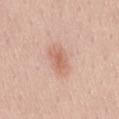No biopsy was performed on this lesion — it was imaged during a full skin examination and was not determined to be concerning. From the lower back. Captured under white-light illumination. A 15 mm close-up extracted from a 3D total-body photography capture. A female subject, aged 53 to 57.